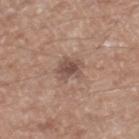Q: Was this lesion biopsied?
A: imaged on a skin check; not biopsied
Q: Where on the body is the lesion?
A: the right lower leg
Q: What are the patient's age and sex?
A: male, aged approximately 65
Q: What is the imaging modality?
A: total-body-photography crop, ~15 mm field of view
Q: What lighting was used for the tile?
A: white-light illumination
Q: What is the lesion's diameter?
A: ~3 mm (longest diameter)
Q: What did automated image analysis measure?
A: an eccentricity of roughly 0.6 and a shape-asymmetry score of about 0.25 (0 = symmetric); a border-irregularity rating of about 2.5/10, a color-variation rating of about 3/10, and a peripheral color-asymmetry measure near 1; an automated nevus-likeness rating near 25 out of 100 and a lesion-detection confidence of about 100/100